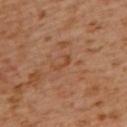Background:
The lesion's longest dimension is about 2.5 mm. The lesion is located on the mid back. Imaged with cross-polarized lighting. The patient is a female about 55 years old. A close-up tile cropped from a whole-body skin photograph, about 15 mm across.